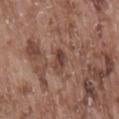No biopsy was performed on this lesion — it was imaged during a full skin examination and was not determined to be concerning. A male subject, aged 73–77. On the lower back. A 15 mm close-up extracted from a 3D total-body photography capture.